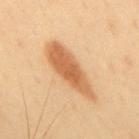Clinical impression:
Recorded during total-body skin imaging; not selected for excision or biopsy.
Background:
The lesion is on the upper back. The tile uses cross-polarized illumination. Approximately 8 mm at its widest. A male subject, in their 40s. This image is a 15 mm lesion crop taken from a total-body photograph.